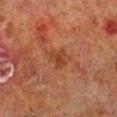{"biopsy_status": "not biopsied; imaged during a skin examination", "patient": {"sex": "male", "age_approx": 70}, "image": {"source": "total-body photography crop", "field_of_view_mm": 15}, "site": "right lower leg", "lesion_size": {"long_diameter_mm_approx": 3.5}}